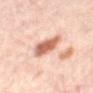Clinical impression: No biopsy was performed on this lesion — it was imaged during a full skin examination and was not determined to be concerning. Clinical summary: A patient aged 53–57. The lesion is on the mid back. This is a cross-polarized tile. A 15 mm crop from a total-body photograph taken for skin-cancer surveillance.